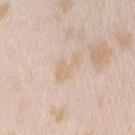Notes:
• workup: no biopsy performed (imaged during a skin exam)
• acquisition: 15 mm crop, total-body photography
• body site: the right upper arm
• patient: female, aged approximately 25
• size: ≈4 mm
• lighting: white-light